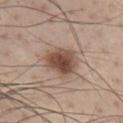biopsy_status: not biopsied; imaged during a skin examination
lighting: white-light
lesion_size:
  long_diameter_mm_approx: 4.0
patient:
  sex: male
  age_approx: 45
site: chest
image:
  source: total-body photography crop
  field_of_view_mm: 15